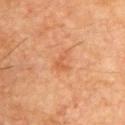Clinical impression:
The lesion was photographed on a routine skin check and not biopsied; there is no pathology result.
Clinical summary:
A male patient aged 58 to 62. On the upper back. The tile uses cross-polarized illumination. A lesion tile, about 15 mm wide, cut from a 3D total-body photograph. Longest diameter approximately 2.5 mm. The lesion-visualizer software estimated a lesion area of about 3 mm², a shape eccentricity near 0.75, and a symmetry-axis asymmetry near 0.5. The analysis additionally found a lesion–skin lightness drop of about 7 and a normalized border contrast of about 5. And it measured a nevus-likeness score of about 5/100 and lesion-presence confidence of about 100/100.